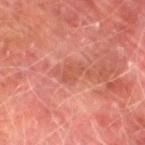Case summary:
– notes: catalogued during a skin exam; not biopsied
– lesion size: ≈2.5 mm
– automated metrics: a footprint of about 3.5 mm², a shape eccentricity near 0.75, and a symmetry-axis asymmetry near 0.35; a color-variation rating of about 1.5/10 and peripheral color asymmetry of about 0.5; a nevus-likeness score of about 0/100 and lesion-presence confidence of about 100/100
– subject: male, roughly 75 years of age
– illumination: cross-polarized illumination
– image: ~15 mm crop, total-body skin-cancer survey
– location: the left lower leg